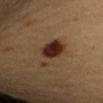Impression: Captured during whole-body skin photography for melanoma surveillance; the lesion was not biopsied. Context: The lesion is located on the right upper arm. This is a cross-polarized tile. This image is a 15 mm lesion crop taken from a total-body photograph. About 6 mm across. The lesion-visualizer software estimated an area of roughly 11 mm² and an eccentricity of roughly 0.9. And it measured a border-irregularity rating of about 4.5/10, internal color variation of about 4.5 on a 0–10 scale, and radial color variation of about 1.5. It also reported an automated nevus-likeness rating near 100 out of 100 and a lesion-detection confidence of about 100/100. A female subject, aged 33 to 37.